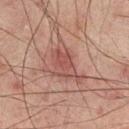  biopsy_status: not biopsied; imaged during a skin examination
  site: back
  patient:
    sex: male
    age_approx: 65
  image:
    source: total-body photography crop
    field_of_view_mm: 15